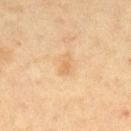workup: imaged on a skin check; not biopsied | site: the leg | acquisition: ~15 mm crop, total-body skin-cancer survey | TBP lesion metrics: a mean CIELAB color near L≈58 a*≈16 b*≈35, roughly 6 lightness units darker than nearby skin, and a lesion-to-skin contrast of about 5 (normalized; higher = more distinct); a border-irregularity rating of about 3.5/10, internal color variation of about 1 on a 0–10 scale, and radial color variation of about 0; an automated nevus-likeness rating near 0 out of 100 and a detector confidence of about 100 out of 100 that the crop contains a lesion | lesion diameter: ≈2.5 mm | subject: female, in their 50s | lighting: cross-polarized.